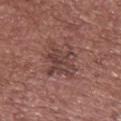Case summary:
- notes · total-body-photography surveillance lesion; no biopsy
- tile lighting · white-light illumination
- automated metrics · an outline eccentricity of about 0.5 (0 = round, 1 = elongated) and a shape-asymmetry score of about 0.25 (0 = symmetric); a mean CIELAB color near L≈42 a*≈21 b*≈22, a lesion–skin lightness drop of about 8, and a normalized lesion–skin contrast near 6.5; border irregularity of about 5 on a 0–10 scale, internal color variation of about 4.5 on a 0–10 scale, and a peripheral color-asymmetry measure near 1.5
- acquisition · total-body-photography crop, ~15 mm field of view
- subject · female, in their mid-60s
- site · the upper back